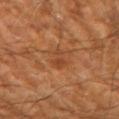• biopsy status — catalogued during a skin exam; not biopsied
• subject — aged 63 to 67
• tile lighting — cross-polarized illumination
• diameter — ≈3 mm
• image — ~15 mm tile from a whole-body skin photo
• anatomic site — the left forearm